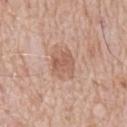biopsy_status: not biopsied; imaged during a skin examination
lighting: white-light
lesion_size:
  long_diameter_mm_approx: 4.0
image:
  source: total-body photography crop
  field_of_view_mm: 15
site: mid back
patient:
  sex: male
  age_approx: 80